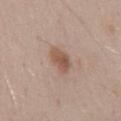Automated image analysis of the tile measured a mean CIELAB color near L≈54 a*≈18 b*≈28 and a normalized border contrast of about 7.5. It also reported a border-irregularity index near 2/10, a color-variation rating of about 3.5/10, and a peripheral color-asymmetry measure near 1. And it measured an automated nevus-likeness rating near 80 out of 100 and a detector confidence of about 100 out of 100 that the crop contains a lesion. A male patient approximately 55 years of age. This is a white-light tile. The lesion is on the mid back. A 15 mm crop from a total-body photograph taken for skin-cancer surveillance.